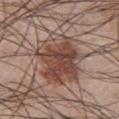Case summary:
– subject: male, in their mid-40s
– acquisition: ~15 mm tile from a whole-body skin photo
– anatomic site: the chest
– lesion diameter: about 7 mm
– lighting: white-light